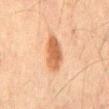Findings:
* biopsy status: imaged on a skin check; not biopsied
* illumination: cross-polarized illumination
* size: ~5 mm (longest diameter)
* site: the abdomen
* patient: male, aged approximately 70
* imaging modality: total-body-photography crop, ~15 mm field of view